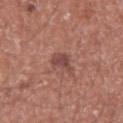Impression:
The lesion was photographed on a routine skin check and not biopsied; there is no pathology result.
Clinical summary:
Automated tile analysis of the lesion measured a lesion area of about 4 mm², a shape eccentricity near 0.9, and a symmetry-axis asymmetry near 0.3. This is a white-light tile. On the right upper arm. The lesion's longest dimension is about 3.5 mm. A male patient, aged 63–67. A close-up tile cropped from a whole-body skin photograph, about 15 mm across.